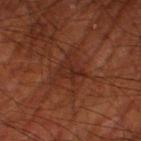This lesion was catalogued during total-body skin photography and was not selected for biopsy. A male subject aged around 70. The tile uses cross-polarized illumination. From the right thigh. The lesion's longest dimension is about 3 mm. A roughly 15 mm field-of-view crop from a total-body skin photograph.